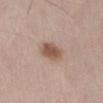The lesion was tiled from a total-body skin photograph and was not biopsied. The tile uses white-light illumination. From the abdomen. An algorithmic analysis of the crop reported a footprint of about 7 mm² and two-axis asymmetry of about 0.15. It also reported an average lesion color of about L≈54 a*≈18 b*≈27 (CIELAB), about 12 CIELAB-L* units darker than the surrounding skin, and a normalized border contrast of about 8.5. It also reported a border-irregularity rating of about 1.5/10, a within-lesion color-variation index near 3/10, and peripheral color asymmetry of about 1. A male subject roughly 60 years of age. A 15 mm close-up extracted from a 3D total-body photography capture. Longest diameter approximately 3.5 mm.